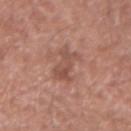Case summary:
– biopsy status · total-body-photography surveillance lesion; no biopsy
– image source · ~15 mm crop, total-body skin-cancer survey
– patient · male, aged approximately 65
– body site · the left forearm
– lesion size · ≈4.5 mm